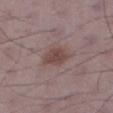Imaged during a routine full-body skin examination; the lesion was not biopsied and no histopathology is available. The lesion's longest dimension is about 3.5 mm. This is a white-light tile. A 15 mm close-up tile from a total-body photography series done for melanoma screening. From the right thigh. A male patient, roughly 50 years of age.